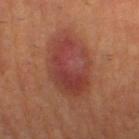Q: Was this lesion biopsied?
A: total-body-photography surveillance lesion; no biopsy
Q: How large is the lesion?
A: ≈8.5 mm
Q: What is the imaging modality?
A: total-body-photography crop, ~15 mm field of view
Q: What lighting was used for the tile?
A: cross-polarized illumination
Q: What is the anatomic site?
A: the right thigh
Q: Who is the patient?
A: male